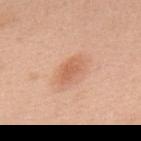Captured during whole-body skin photography for melanoma surveillance; the lesion was not biopsied. Automated tile analysis of the lesion measured a lesion color around L≈62 a*≈24 b*≈34 in CIELAB, a lesion–skin lightness drop of about 9, and a lesion-to-skin contrast of about 6 (normalized; higher = more distinct). The software also gave a classifier nevus-likeness of about 85/100 and a detector confidence of about 100 out of 100 that the crop contains a lesion. This is a white-light tile. The subject is a male approximately 70 years of age. Longest diameter approximately 3.5 mm. A lesion tile, about 15 mm wide, cut from a 3D total-body photograph. The lesion is located on the upper back.